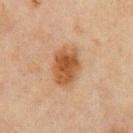  biopsy_status: not biopsied; imaged during a skin examination
  image:
    source: total-body photography crop
    field_of_view_mm: 15
  lighting: cross-polarized
  patient:
    sex: male
    age_approx: 45
  lesion_size:
    long_diameter_mm_approx: 5.0
  automated_metrics:
    cielab_L: 43
    cielab_a: 19
    cielab_b: 32
    vs_skin_contrast_norm: 9.5
    border_irregularity_0_10: 2.0
    color_variation_0_10: 4.0
    peripheral_color_asymmetry: 1.5
    nevus_likeness_0_100: 95
    lesion_detection_confidence_0_100: 100
  site: chest